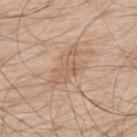No biopsy was performed on this lesion — it was imaged during a full skin examination and was not determined to be concerning.
A male patient roughly 70 years of age.
A 15 mm close-up extracted from a 3D total-body photography capture.
The lesion is on the back.